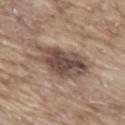This lesion was catalogued during total-body skin photography and was not selected for biopsy.
Automated image analysis of the tile measured an area of roughly 20 mm², a shape eccentricity near 0.85, and a shape-asymmetry score of about 0.25 (0 = symmetric). The analysis additionally found an average lesion color of about L≈48 a*≈15 b*≈23 (CIELAB) and about 13 CIELAB-L* units darker than the surrounding skin. The analysis additionally found a border-irregularity index near 4/10 and a peripheral color-asymmetry measure near 2.5. It also reported a detector confidence of about 90 out of 100 that the crop contains a lesion.
The subject is a male aged 63–67.
The tile uses white-light illumination.
Measured at roughly 7.5 mm in maximum diameter.
This image is a 15 mm lesion crop taken from a total-body photograph.
The lesion is located on the back.